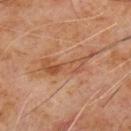Assessment:
Recorded during total-body skin imaging; not selected for excision or biopsy.
Image and clinical context:
The recorded lesion diameter is about 6.5 mm. A male patient aged around 60. Captured under cross-polarized illumination. This image is a 15 mm lesion crop taken from a total-body photograph. The lesion is on the chest. Automated tile analysis of the lesion measured a footprint of about 9.5 mm², a shape eccentricity near 0.95, and a shape-asymmetry score of about 0.55 (0 = symmetric).